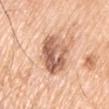Recorded during total-body skin imaging; not selected for excision or biopsy. The subject is a male in their mid-50s. The lesion is located on the left upper arm. The tile uses white-light illumination. Approximately 5.5 mm at its widest. Automated image analysis of the tile measured an automated nevus-likeness rating near 20 out of 100 and a detector confidence of about 100 out of 100 that the crop contains a lesion. Cropped from a whole-body photographic skin survey; the tile spans about 15 mm.